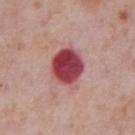Imaged during a routine full-body skin examination; the lesion was not biopsied and no histopathology is available.